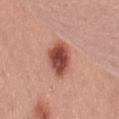Imaged during a routine full-body skin examination; the lesion was not biopsied and no histopathology is available. Imaged with white-light lighting. A 15 mm close-up extracted from a 3D total-body photography capture. The total-body-photography lesion software estimated a lesion area of about 10 mm², an outline eccentricity of about 0.75 (0 = round, 1 = elongated), and a symmetry-axis asymmetry near 0.15. And it measured an average lesion color of about L≈49 a*≈28 b*≈28 (CIELAB) and a lesion–skin lightness drop of about 17. The analysis additionally found border irregularity of about 1.5 on a 0–10 scale, internal color variation of about 5.5 on a 0–10 scale, and a peripheral color-asymmetry measure near 1.5. The recorded lesion diameter is about 4.5 mm. The patient is a female aged approximately 25. The lesion is on the lower back.